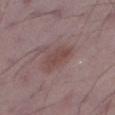Clinical impression: No biopsy was performed on this lesion — it was imaged during a full skin examination and was not determined to be concerning. Image and clinical context: Located on the right thigh. A male patient, aged 48–52. This image is a 15 mm lesion crop taken from a total-body photograph.